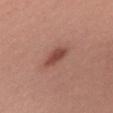Assessment:
Imaged during a routine full-body skin examination; the lesion was not biopsied and no histopathology is available.
Acquisition and patient details:
Located on the upper back. A lesion tile, about 15 mm wide, cut from a 3D total-body photograph. A female patient about 20 years old.